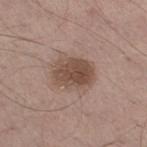| key | value |
|---|---|
| follow-up | total-body-photography surveillance lesion; no biopsy |
| automated lesion analysis | a mean CIELAB color near L≈48 a*≈17 b*≈25, a lesion–skin lightness drop of about 11, and a normalized lesion–skin contrast near 8.5; a border-irregularity index near 2/10, a color-variation rating of about 4/10, and radial color variation of about 1.5; a classifier nevus-likeness of about 25/100 |
| lighting | white-light illumination |
| patient | male, aged around 60 |
| diameter | ~4 mm (longest diameter) |
| location | the right thigh |
| acquisition | total-body-photography crop, ~15 mm field of view |